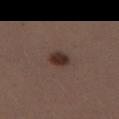Assessment:
No biopsy was performed on this lesion — it was imaged during a full skin examination and was not determined to be concerning.
Clinical summary:
A close-up tile cropped from a whole-body skin photograph, about 15 mm across. The subject is a female aged approximately 40. Automated tile analysis of the lesion measured a border-irregularity rating of about 1.5/10, a within-lesion color-variation index near 3/10, and radial color variation of about 1. The lesion is located on the leg.